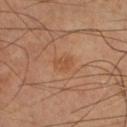Case summary:
- imaging modality · ~15 mm tile from a whole-body skin photo
- size · ≈2.5 mm
- subject · male, aged approximately 70
- TBP lesion metrics · a mean CIELAB color near L≈49 a*≈22 b*≈34 and a normalized border contrast of about 5.5; a border-irregularity index near 2.5/10 and internal color variation of about 2 on a 0–10 scale
- body site · the right lower leg
- illumination · cross-polarized illumination